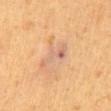The subject is a male about 55 years old.
On the abdomen.
A close-up tile cropped from a whole-body skin photograph, about 15 mm across.
The tile uses cross-polarized illumination.